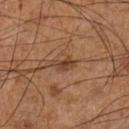No biopsy was performed on this lesion — it was imaged during a full skin examination and was not determined to be concerning. A male subject, about 60 years old. This image is a 15 mm lesion crop taken from a total-body photograph. Located on the left lower leg. Longest diameter approximately 2.5 mm. Captured under cross-polarized illumination.